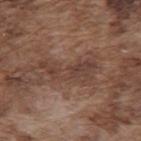Captured during whole-body skin photography for melanoma surveillance; the lesion was not biopsied. The lesion-visualizer software estimated a footprint of about 11 mm², an eccentricity of roughly 0.95, and a symmetry-axis asymmetry near 0.45. From the back. A male patient, in their mid-70s. Measured at roughly 6.5 mm in maximum diameter. This image is a 15 mm lesion crop taken from a total-body photograph. This is a white-light tile.